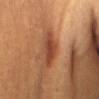biopsy status: no biopsy performed (imaged during a skin exam); image source: total-body-photography crop, ~15 mm field of view; lighting: cross-polarized; anatomic site: the abdomen; patient: female, roughly 30 years of age; size: ~4.5 mm (longest diameter).